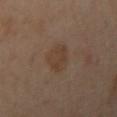The lesion was tiled from a total-body skin photograph and was not biopsied.
A female subject aged approximately 40.
Imaged with cross-polarized lighting.
About 3.5 mm across.
A 15 mm close-up extracted from a 3D total-body photography capture.
On the left arm.